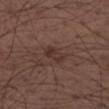{"biopsy_status": "not biopsied; imaged during a skin examination", "patient": {"sex": "male", "age_approx": 40}, "site": "arm", "lesion_size": {"long_diameter_mm_approx": 3.0}, "image": {"source": "total-body photography crop", "field_of_view_mm": 15}}